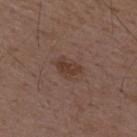notes = total-body-photography surveillance lesion; no biopsy | site = the upper back | diameter = ≈3.5 mm | illumination = white-light | acquisition = 15 mm crop, total-body photography | patient = male, aged 48 to 52 | automated lesion analysis = border irregularity of about 3 on a 0–10 scale and peripheral color asymmetry of about 1; a nevus-likeness score of about 70/100 and a lesion-detection confidence of about 100/100.